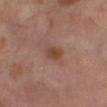Findings:
* follow-up: imaged on a skin check; not biopsied
* location: the right thigh
* acquisition: ~15 mm crop, total-body skin-cancer survey
* illumination: cross-polarized illumination
* diameter: about 2.5 mm
* patient: female, aged 53–57
* TBP lesion metrics: a lesion color around L≈45 a*≈21 b*≈28 in CIELAB and a lesion-to-skin contrast of about 7.5 (normalized; higher = more distinct); a nevus-likeness score of about 60/100 and lesion-presence confidence of about 100/100